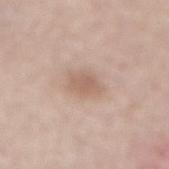– biopsy status — imaged on a skin check; not biopsied
– lesion size — ≈3 mm
– image source — total-body-photography crop, ~15 mm field of view
– illumination — white-light
– anatomic site — the lower back
– subject — male, aged 78 to 82